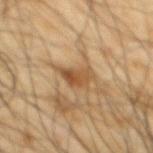notes — catalogued during a skin exam; not biopsied
TBP lesion metrics — a normalized lesion–skin contrast near 7.5
body site — the mid back
lighting — cross-polarized illumination
patient — male, aged 63–67
image — ~15 mm tile from a whole-body skin photo
lesion diameter — about 3.5 mm